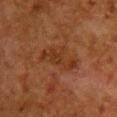Part of a total-body skin-imaging series; this lesion was reviewed on a skin check and was not flagged for biopsy. The lesion's longest dimension is about 5 mm. The subject is a female in their 50s. This is a cross-polarized tile. Automated image analysis of the tile measured an area of roughly 10 mm² and an outline eccentricity of about 0.85 (0 = round, 1 = elongated). It also reported a border-irregularity rating of about 4.5/10, a color-variation rating of about 2.5/10, and a peripheral color-asymmetry measure near 0.5. The software also gave a nevus-likeness score of about 0/100 and a lesion-detection confidence of about 100/100. Located on the chest. A lesion tile, about 15 mm wide, cut from a 3D total-body photograph.